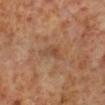Findings:
- biopsy status · total-body-photography surveillance lesion; no biopsy
- subject · male, in their 60s
- illumination · cross-polarized illumination
- site · the leg
- acquisition · 15 mm crop, total-body photography